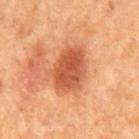follow-up: no biopsy performed (imaged during a skin exam) | subject: male, approximately 65 years of age | body site: the mid back | lesion diameter: ~6 mm (longest diameter) | image: 15 mm crop, total-body photography.